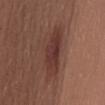Context:
On the mid back. A 15 mm crop from a total-body photograph taken for skin-cancer surveillance. A female patient, aged around 25.